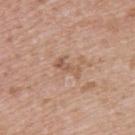<case>
  <site>upper back</site>
  <patient>
    <sex>female</sex>
    <age_approx>40</age_approx>
  </patient>
  <image>
    <source>total-body photography crop</source>
    <field_of_view_mm>15</field_of_view_mm>
  </image>
</case>